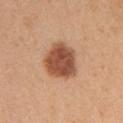biopsy_status: not biopsied; imaged during a skin examination
patient:
  sex: female
  age_approx: 45
lesion_size:
  long_diameter_mm_approx: 4.5
site: arm
image:
  source: total-body photography crop
  field_of_view_mm: 15
lighting: white-light